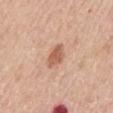The subject is a male aged 58 to 62. A close-up tile cropped from a whole-body skin photograph, about 15 mm across. The lesion is located on the mid back.